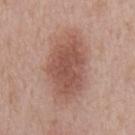Notes:
• biopsy status: total-body-photography surveillance lesion; no biopsy
• diameter: ≈8 mm
• body site: the chest
• image source: 15 mm crop, total-body photography
• illumination: white-light
• subject: male, aged around 65